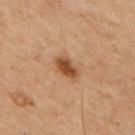Clinical impression: Recorded during total-body skin imaging; not selected for excision or biopsy. Context: The total-body-photography lesion software estimated a lesion area of about 5.5 mm² and an outline eccentricity of about 0.6 (0 = round, 1 = elongated). The analysis additionally found a lesion color around L≈36 a*≈18 b*≈28 in CIELAB and a normalized border contrast of about 9.5. It also reported internal color variation of about 3.5 on a 0–10 scale and a peripheral color-asymmetry measure near 1. A male subject aged approximately 70. The lesion is located on the right upper arm. About 3 mm across. A lesion tile, about 15 mm wide, cut from a 3D total-body photograph. The tile uses cross-polarized illumination.